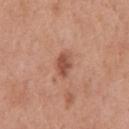Imaged during a routine full-body skin examination; the lesion was not biopsied and no histopathology is available.
The lesion-visualizer software estimated an area of roughly 4.5 mm², an eccentricity of roughly 0.8, and two-axis asymmetry of about 0.25. And it measured an average lesion color of about L≈51 a*≈25 b*≈30 (CIELAB), about 11 CIELAB-L* units darker than the surrounding skin, and a normalized border contrast of about 8. The software also gave a border-irregularity index near 2.5/10.
Cropped from a whole-body photographic skin survey; the tile spans about 15 mm.
A male subject, roughly 70 years of age.
The lesion's longest dimension is about 3 mm.
The lesion is on the chest.